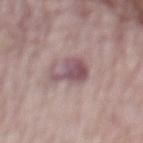illumination: white-light illumination | anatomic site: the mid back | patient: male, approximately 65 years of age | image: ~15 mm crop, total-body skin-cancer survey | lesion size: about 4 mm.